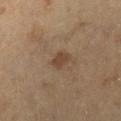biopsy status = imaged on a skin check; not biopsied | illumination = cross-polarized illumination | patient = about 60 years old | diameter = ≈2.5 mm | anatomic site = the right lower leg | image = 15 mm crop, total-body photography.